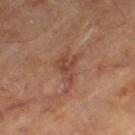This lesion was catalogued during total-body skin photography and was not selected for biopsy.
Automated image analysis of the tile measured a lesion area of about 8.5 mm² and a shape-asymmetry score of about 0.5 (0 = symmetric). It also reported a border-irregularity rating of about 6.5/10, a within-lesion color-variation index near 3.5/10, and radial color variation of about 1.
Measured at roughly 5 mm in maximum diameter.
The lesion is located on the leg.
A subject in their mid- to late 60s.
This is a cross-polarized tile.
A region of skin cropped from a whole-body photographic capture, roughly 15 mm wide.